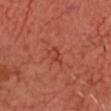Captured during whole-body skin photography for melanoma surveillance; the lesion was not biopsied. Approximately 3 mm at its widest. Located on the head or neck. The patient is approximately 65 years of age. An algorithmic analysis of the crop reported roughly 6 lightness units darker than nearby skin. This is a cross-polarized tile. Cropped from a whole-body photographic skin survey; the tile spans about 15 mm.